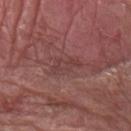Q: Is there a histopathology result?
A: total-body-photography surveillance lesion; no biopsy
Q: What are the patient's age and sex?
A: male, aged 78–82
Q: What kind of image is this?
A: total-body-photography crop, ~15 mm field of view
Q: What did automated image analysis measure?
A: an average lesion color of about L≈40 a*≈23 b*≈20 (CIELAB), roughly 6 lightness units darker than nearby skin, and a normalized border contrast of about 5; a nevus-likeness score of about 0/100
Q: Illumination type?
A: white-light illumination
Q: What is the lesion's diameter?
A: about 3.5 mm
Q: What is the anatomic site?
A: the left forearm